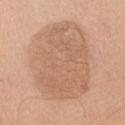notes — total-body-photography surveillance lesion; no biopsy | lesion diameter — ≈9.5 mm | subject — male, about 60 years old | tile lighting — white-light | image-analysis metrics — a footprint of about 55 mm² and a shape-asymmetry score of about 0.1 (0 = symmetric); a lesion color around L≈62 a*≈19 b*≈32 in CIELAB and roughly 8 lightness units darker than nearby skin | acquisition — ~15 mm crop, total-body skin-cancer survey | location — the chest.